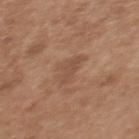No biopsy was performed on this lesion — it was imaged during a full skin examination and was not determined to be concerning. The patient is a female aged approximately 40. Cropped from a total-body skin-imaging series; the visible field is about 15 mm. The lesion is located on the upper back.